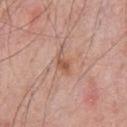A male patient in their 60s.
On the chest.
Captured under white-light illumination.
Measured at roughly 3 mm in maximum diameter.
Cropped from a total-body skin-imaging series; the visible field is about 15 mm.